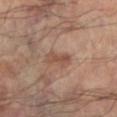Assessment: Imaged during a routine full-body skin examination; the lesion was not biopsied and no histopathology is available. Acquisition and patient details: A male patient, approximately 60 years of age. Cropped from a whole-body photographic skin survey; the tile spans about 15 mm. An algorithmic analysis of the crop reported border irregularity of about 3.5 on a 0–10 scale, a within-lesion color-variation index near 0/10, and radial color variation of about 0. The tile uses cross-polarized illumination. On the left lower leg. The recorded lesion diameter is about 3 mm.